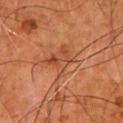Q: Is there a histopathology result?
A: no biopsy performed (imaged during a skin exam)
Q: Lesion location?
A: the front of the torso
Q: What lighting was used for the tile?
A: cross-polarized illumination
Q: What is the lesion's diameter?
A: ≈4.5 mm
Q: How was this image acquired?
A: total-body-photography crop, ~15 mm field of view
Q: Who is the patient?
A: male, aged 53 to 57